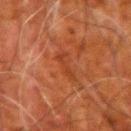Imaged during a routine full-body skin examination; the lesion was not biopsied and no histopathology is available. A male patient, aged 78–82. Located on the left upper arm. Measured at roughly 3.5 mm in maximum diameter. This is a cross-polarized tile. An algorithmic analysis of the crop reported an outline eccentricity of about 0.9 (0 = round, 1 = elongated) and a symmetry-axis asymmetry near 0.55. And it measured a border-irregularity index near 6.5/10, internal color variation of about 0 on a 0–10 scale, and peripheral color asymmetry of about 0. And it measured a classifier nevus-likeness of about 0/100 and a detector confidence of about 100 out of 100 that the crop contains a lesion. A 15 mm close-up extracted from a 3D total-body photography capture.